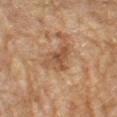Findings:
• workup: no biopsy performed (imaged during a skin exam)
• illumination: cross-polarized
• image source: ~15 mm tile from a whole-body skin photo
• site: the left forearm
• subject: female, aged 78 to 82
• automated lesion analysis: a lesion area of about 8.5 mm² and two-axis asymmetry of about 0.45; a lesion color around L≈43 a*≈17 b*≈29 in CIELAB, a lesion–skin lightness drop of about 8, and a normalized border contrast of about 6.5; border irregularity of about 5 on a 0–10 scale, internal color variation of about 4 on a 0–10 scale, and peripheral color asymmetry of about 1